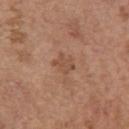{"biopsy_status": "not biopsied; imaged during a skin examination", "patient": {"sex": "female", "age_approx": 65}, "site": "left upper arm", "lighting": "white-light", "lesion_size": {"long_diameter_mm_approx": 2.5}, "image": {"source": "total-body photography crop", "field_of_view_mm": 15}}